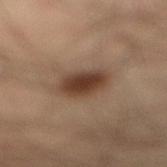Impression: This lesion was catalogued during total-body skin photography and was not selected for biopsy. Image and clinical context: This image is a 15 mm lesion crop taken from a total-body photograph. Approximately 4 mm at its widest. A male subject aged around 35. Located on the right lower leg.